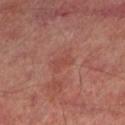The lesion was photographed on a routine skin check and not biopsied; there is no pathology result.
From the left lower leg.
Cropped from a total-body skin-imaging series; the visible field is about 15 mm.
A male patient, roughly 70 years of age.
The recorded lesion diameter is about 2.5 mm.
The lesion-visualizer software estimated an area of roughly 3 mm², an eccentricity of roughly 0.85, and a shape-asymmetry score of about 0.4 (0 = symmetric). The analysis additionally found a lesion color around L≈44 a*≈26 b*≈26 in CIELAB, roughly 5 lightness units darker than nearby skin, and a normalized border contrast of about 4.5. The analysis additionally found a border-irregularity rating of about 4.5/10.